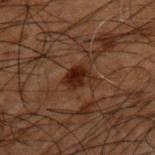Imaged during a routine full-body skin examination; the lesion was not biopsied and no histopathology is available.
A male patient aged around 50.
Longest diameter approximately 3 mm.
Imaged with cross-polarized lighting.
A lesion tile, about 15 mm wide, cut from a 3D total-body photograph.
The total-body-photography lesion software estimated a lesion area of about 5 mm² and a shape-asymmetry score of about 0.15 (0 = symmetric). The analysis additionally found a mean CIELAB color near L≈18 a*≈18 b*≈20, roughly 9 lightness units darker than nearby skin, and a normalized border contrast of about 11. The analysis additionally found a classifier nevus-likeness of about 95/100 and lesion-presence confidence of about 100/100.
On the upper back.